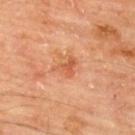| key | value |
|---|---|
| body site | the upper back |
| illumination | cross-polarized |
| automated lesion analysis | a lesion area of about 4 mm², an outline eccentricity of about 0.6 (0 = round, 1 = elongated), and two-axis asymmetry of about 0.55; an average lesion color of about L≈57 a*≈28 b*≈39 (CIELAB) and a normalized lesion–skin contrast near 6; an automated nevus-likeness rating near 5 out of 100 and lesion-presence confidence of about 100/100 |
| acquisition | 15 mm crop, total-body photography |
| patient | male, aged 63–67 |
| diameter | ≈3 mm |